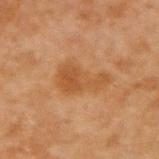An algorithmic analysis of the crop reported an automated nevus-likeness rating near 10 out of 100.
The tile uses cross-polarized illumination.
A roughly 15 mm field-of-view crop from a total-body skin photograph.
The subject is a male aged 43–47.
Longest diameter approximately 5 mm.
Located on the right upper arm.